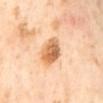The lesion was tiled from a total-body skin photograph and was not biopsied. About 4.5 mm across. The lesion-visualizer software estimated a border-irregularity rating of about 2.5/10, internal color variation of about 6 on a 0–10 scale, and peripheral color asymmetry of about 2. The analysis additionally found a nevus-likeness score of about 65/100 and a lesion-detection confidence of about 100/100. A female patient aged 53–57. Cropped from a whole-body photographic skin survey; the tile spans about 15 mm. From the mid back.